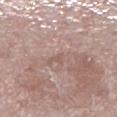lighting: white-light
automated_metrics:
  cielab_L: 56
  cielab_a: 18
  cielab_b: 22
  vs_skin_darker_L: 5.0
  border_irregularity_0_10: 3.0
  peripheral_color_asymmetry: 0.0
site: right lower leg
image:
  source: total-body photography crop
  field_of_view_mm: 15
patient:
  sex: female
  age_approx: 60